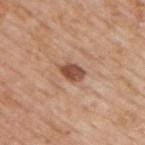Part of a total-body skin-imaging series; this lesion was reviewed on a skin check and was not flagged for biopsy.
The patient is a male in their mid- to late 70s.
The tile uses white-light illumination.
A 15 mm crop from a total-body photograph taken for skin-cancer surveillance.
From the upper back.
The total-body-photography lesion software estimated an area of roughly 4.5 mm², an outline eccentricity of about 0.75 (0 = round, 1 = elongated), and a shape-asymmetry score of about 0.2 (0 = symmetric). The analysis additionally found an average lesion color of about L≈50 a*≈23 b*≈30 (CIELAB), a lesion–skin lightness drop of about 14, and a normalized border contrast of about 9.5. It also reported an automated nevus-likeness rating near 75 out of 100 and a detector confidence of about 100 out of 100 that the crop contains a lesion.
Approximately 3 mm at its widest.